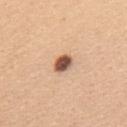Captured during whole-body skin photography for melanoma surveillance; the lesion was not biopsied. A 15 mm close-up extracted from a 3D total-body photography capture. The tile uses white-light illumination. The recorded lesion diameter is about 2.5 mm. Automated tile analysis of the lesion measured a shape eccentricity near 0.65 and a symmetry-axis asymmetry near 0.2. It also reported lesion-presence confidence of about 100/100. From the upper back. A female subject aged approximately 45.